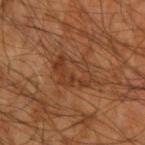Image and clinical context:
Longest diameter approximately 5.5 mm. The lesion is located on the left arm. The subject is a male roughly 60 years of age. The tile uses cross-polarized illumination. The lesion-visualizer software estimated a mean CIELAB color near L≈37 a*≈22 b*≈32, about 6 CIELAB-L* units darker than the surrounding skin, and a normalized lesion–skin contrast near 5.5. A close-up tile cropped from a whole-body skin photograph, about 15 mm across.